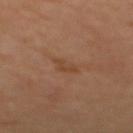Imaged during a routine full-body skin examination; the lesion was not biopsied and no histopathology is available. A 15 mm crop from a total-body photograph taken for skin-cancer surveillance. A female patient aged 38 to 42. The lesion-visualizer software estimated a mean CIELAB color near L≈43 a*≈20 b*≈32, a lesion–skin lightness drop of about 6, and a normalized lesion–skin contrast near 5.5. And it measured a classifier nevus-likeness of about 0/100 and a detector confidence of about 100 out of 100 that the crop contains a lesion. The lesion is located on the upper back.